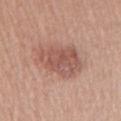follow-up: catalogued during a skin exam; not biopsied | automated metrics: a footprint of about 18 mm² and a shape eccentricity near 0.75; a lesion color around L≈54 a*≈22 b*≈26 in CIELAB, about 10 CIELAB-L* units darker than the surrounding skin, and a lesion-to-skin contrast of about 7 (normalized; higher = more distinct); a border-irregularity index near 3/10, a color-variation rating of about 4.5/10, and radial color variation of about 1.5 | image: 15 mm crop, total-body photography | diameter: ~6 mm (longest diameter) | tile lighting: white-light illumination | subject: male, approximately 55 years of age | location: the right upper arm.